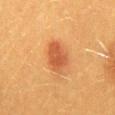Findings:
- workup: no biopsy performed (imaged during a skin exam)